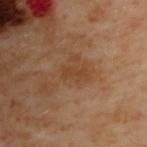{"biopsy_status": "not biopsied; imaged during a skin examination", "site": "back", "image": {"source": "total-body photography crop", "field_of_view_mm": 15}, "patient": {"sex": "female", "age_approx": 60}}